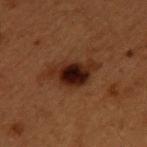This lesion was catalogued during total-body skin photography and was not selected for biopsy.
On the upper back.
A male patient aged around 50.
Imaged with cross-polarized lighting.
Approximately 5 mm at its widest.
A lesion tile, about 15 mm wide, cut from a 3D total-body photograph.